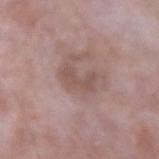Impression: The lesion was tiled from a total-body skin photograph and was not biopsied. Acquisition and patient details: Cropped from a total-body skin-imaging series; the visible field is about 15 mm. Longest diameter approximately 4 mm. This is a white-light tile. A male subject roughly 65 years of age. On the left forearm.